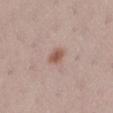The lesion was tiled from a total-body skin photograph and was not biopsied. Captured under white-light illumination. An algorithmic analysis of the crop reported a footprint of about 4 mm². And it measured a border-irregularity index near 2/10, internal color variation of about 2.5 on a 0–10 scale, and a peripheral color-asymmetry measure near 1. From the left lower leg. A region of skin cropped from a whole-body photographic capture, roughly 15 mm wide. About 2.5 mm across. A female subject roughly 25 years of age.